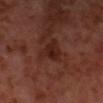biopsy status: total-body-photography surveillance lesion; no biopsy
patient: male, aged approximately 55
lesion diameter: ≈4.5 mm
lighting: cross-polarized illumination
imaging modality: ~15 mm tile from a whole-body skin photo
anatomic site: the head or neck